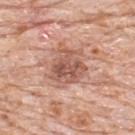notes=catalogued during a skin exam; not biopsied | image source=~15 mm tile from a whole-body skin photo | location=the upper back | image-analysis metrics=an average lesion color of about L≈56 a*≈23 b*≈28 (CIELAB) and roughly 11 lightness units darker than nearby skin; a border-irregularity rating of about 3.5/10, a color-variation rating of about 5.5/10, and a peripheral color-asymmetry measure near 2; a detector confidence of about 100 out of 100 that the crop contains a lesion | patient=male, aged 78 to 82 | lesion size=about 4.5 mm | lighting=white-light.